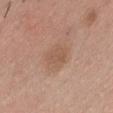Assessment: No biopsy was performed on this lesion — it was imaged during a full skin examination and was not determined to be concerning. Background: A lesion tile, about 15 mm wide, cut from a 3D total-body photograph. A male patient, aged 28–32. Located on the front of the torso.